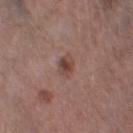Background:
A male patient in their mid-70s. This is a white-light tile. A region of skin cropped from a whole-body photographic capture, roughly 15 mm wide. Approximately 3 mm at its widest. On the leg. Automated tile analysis of the lesion measured a mean CIELAB color near L≈44 a*≈20 b*≈23, roughly 9 lightness units darker than nearby skin, and a lesion-to-skin contrast of about 7 (normalized; higher = more distinct). And it measured a nevus-likeness score of about 75/100 and a lesion-detection confidence of about 100/100.